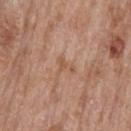workup: catalogued during a skin exam; not biopsied | site: the mid back | imaging modality: ~15 mm tile from a whole-body skin photo | lesion size: about 2.5 mm | subject: male, aged 63–67 | illumination: white-light illumination.